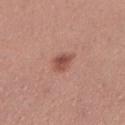This lesion was catalogued during total-body skin photography and was not selected for biopsy. Located on the left lower leg. A lesion tile, about 15 mm wide, cut from a 3D total-body photograph. A female subject aged 28–32.